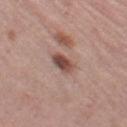| feature | finding |
|---|---|
| workup | imaged on a skin check; not biopsied |
| site | the right thigh |
| lighting | white-light illumination |
| TBP lesion metrics | a footprint of about 5 mm², an outline eccentricity of about 0.65 (0 = round, 1 = elongated), and two-axis asymmetry of about 0.2; an average lesion color of about L≈48 a*≈20 b*≈24 (CIELAB), roughly 13 lightness units darker than nearby skin, and a normalized lesion–skin contrast near 9.5; border irregularity of about 2 on a 0–10 scale and a color-variation rating of about 4.5/10 |
| imaging modality | total-body-photography crop, ~15 mm field of view |
| patient | female, approximately 40 years of age |
| lesion diameter | about 2.5 mm |